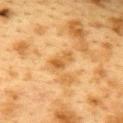{"biopsy_status": "not biopsied; imaged during a skin examination", "image": {"source": "total-body photography crop", "field_of_view_mm": 15}, "patient": {"sex": "female", "age_approx": 40}, "site": "upper back"}